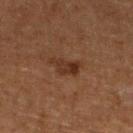Findings:
* biopsy status: catalogued during a skin exam; not biopsied
* image-analysis metrics: a nevus-likeness score of about 40/100 and a lesion-detection confidence of about 100/100
* diameter: ~4 mm (longest diameter)
* patient: male, roughly 75 years of age
* image: total-body-photography crop, ~15 mm field of view
* body site: the left lower leg